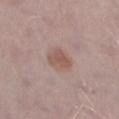{"lighting": "white-light", "site": "left thigh", "patient": {"sex": "male", "age_approx": 75}, "image": {"source": "total-body photography crop", "field_of_view_mm": 15}, "lesion_size": {"long_diameter_mm_approx": 2.5}}